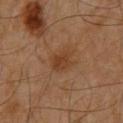Case summary:
* workup — catalogued during a skin exam; not biopsied
* subject — male, aged 58 to 62
* lighting — cross-polarized
* imaging modality — ~15 mm crop, total-body skin-cancer survey
* body site — the chest
* TBP lesion metrics — a mean CIELAB color near L≈35 a*≈20 b*≈30, roughly 7 lightness units darker than nearby skin, and a normalized lesion–skin contrast near 6.5; a classifier nevus-likeness of about 55/100 and a lesion-detection confidence of about 100/100